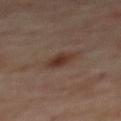Q: Was a biopsy performed?
A: catalogued during a skin exam; not biopsied
Q: Lesion location?
A: the mid back
Q: Lesion size?
A: ≈3 mm
Q: What are the patient's age and sex?
A: male, approximately 70 years of age
Q: What kind of image is this?
A: 15 mm crop, total-body photography